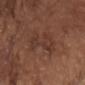Recorded during total-body skin imaging; not selected for excision or biopsy. A region of skin cropped from a whole-body photographic capture, roughly 15 mm wide. The patient is a male aged around 75. Imaged with white-light lighting. From the front of the torso.